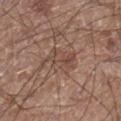Captured during whole-body skin photography for melanoma surveillance; the lesion was not biopsied. Located on the right lower leg. The subject is a male about 60 years old. Measured at roughly 5 mm in maximum diameter. A region of skin cropped from a whole-body photographic capture, roughly 15 mm wide. This is a white-light tile.